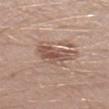Impression: Part of a total-body skin-imaging series; this lesion was reviewed on a skin check and was not flagged for biopsy. Acquisition and patient details: This is a white-light tile. The lesion is on the right lower leg. Longest diameter approximately 5.5 mm. A region of skin cropped from a whole-body photographic capture, roughly 15 mm wide. A male patient aged approximately 30.